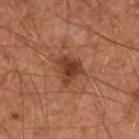No biopsy was performed on this lesion — it was imaged during a full skin examination and was not determined to be concerning.
Located on the right forearm.
A roughly 15 mm field-of-view crop from a total-body skin photograph.
A male patient roughly 45 years of age.